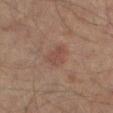biopsy status: catalogued during a skin exam; not biopsied
location: the right thigh
image source: ~15 mm crop, total-body skin-cancer survey
subject: male, approximately 65 years of age
tile lighting: cross-polarized illumination
lesion size: ~3 mm (longest diameter)